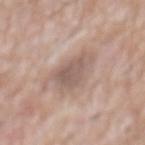Impression: This lesion was catalogued during total-body skin photography and was not selected for biopsy. Background: Imaged with white-light lighting. On the chest. Cropped from a total-body skin-imaging series; the visible field is about 15 mm. A male subject aged 58–62.